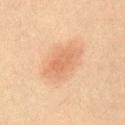Captured during whole-body skin photography for melanoma surveillance; the lesion was not biopsied. A close-up tile cropped from a whole-body skin photograph, about 15 mm across. A male patient, approximately 60 years of age. About 5.5 mm across. Located on the front of the torso. Automated image analysis of the tile measured an area of roughly 15 mm², a shape eccentricity near 0.8, and a symmetry-axis asymmetry near 0.15. The analysis additionally found roughly 7 lightness units darker than nearby skin and a lesion-to-skin contrast of about 5.5 (normalized; higher = more distinct). It also reported a border-irregularity index near 2.5/10 and a within-lesion color-variation index near 2.5/10.